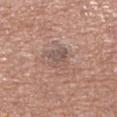Assessment:
No biopsy was performed on this lesion — it was imaged during a full skin examination and was not determined to be concerning.
Image and clinical context:
From the leg. Automated tile analysis of the lesion measured a lesion area of about 6.5 mm² and a symmetry-axis asymmetry near 0.45. Imaged with white-light lighting. A roughly 15 mm field-of-view crop from a total-body skin photograph. A male subject, roughly 75 years of age.